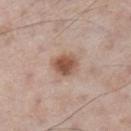Imaged during a routine full-body skin examination; the lesion was not biopsied and no histopathology is available.
The lesion-visualizer software estimated a lesion area of about 7.5 mm², an outline eccentricity of about 0.55 (0 = round, 1 = elongated), and a shape-asymmetry score of about 0.25 (0 = symmetric). The analysis additionally found a lesion color around L≈54 a*≈19 b*≈28 in CIELAB and a normalized border contrast of about 9. The software also gave a border-irregularity index near 2/10, a color-variation rating of about 4.5/10, and peripheral color asymmetry of about 1.5.
On the left lower leg.
This is a white-light tile.
Cropped from a whole-body photographic skin survey; the tile spans about 15 mm.
The recorded lesion diameter is about 3.5 mm.
A male patient, about 60 years old.